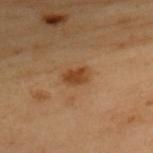No biopsy was performed on this lesion — it was imaged during a full skin examination and was not determined to be concerning.
A close-up tile cropped from a whole-body skin photograph, about 15 mm across.
Automated tile analysis of the lesion measured an area of roughly 4 mm² and two-axis asymmetry of about 0.2. The software also gave an average lesion color of about L≈37 a*≈19 b*≈33 (CIELAB) and a lesion–skin lightness drop of about 9. The analysis additionally found an automated nevus-likeness rating near 60 out of 100 and a lesion-detection confidence of about 100/100.
A female patient aged 58 to 62.
About 2.5 mm across.
From the upper back.